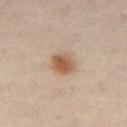– workup: imaged on a skin check; not biopsied
– lighting: cross-polarized illumination
– image: ~15 mm tile from a whole-body skin photo
– size: ≈3 mm
– location: the left leg
– TBP lesion metrics: roughly 12 lightness units darker than nearby skin and a normalized lesion–skin contrast near 9; a nevus-likeness score of about 100/100 and lesion-presence confidence of about 100/100
– patient: female, in their 40s